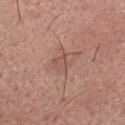Findings:
– lighting — white-light illumination
– anatomic site — the head or neck
– acquisition — ~15 mm tile from a whole-body skin photo
– patient — male, in their 70s